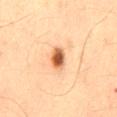Clinical impression:
Part of a total-body skin-imaging series; this lesion was reviewed on a skin check and was not flagged for biopsy.
Acquisition and patient details:
The lesion is located on the lower back. A 15 mm crop from a total-body photograph taken for skin-cancer surveillance. The subject is a male about 65 years old.